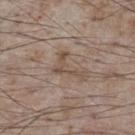automated lesion analysis = an eccentricity of roughly 0.85; a mean CIELAB color near L≈50 a*≈14 b*≈25, a lesion–skin lightness drop of about 7, and a normalized border contrast of about 6; a lesion-detection confidence of about 80/100 | imaging modality = ~15 mm tile from a whole-body skin photo | tile lighting = white-light illumination | anatomic site = the chest | lesion size = ≈4 mm | patient = male, roughly 75 years of age.